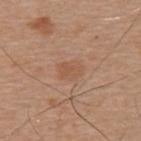follow-up: imaged on a skin check; not biopsied | patient: male, aged 63–67 | imaging modality: total-body-photography crop, ~15 mm field of view | illumination: white-light | TBP lesion metrics: a lesion area of about 5 mm² and an eccentricity of roughly 0.7; an average lesion color of about L≈54 a*≈21 b*≈32 (CIELAB), roughly 6 lightness units darker than nearby skin, and a normalized border contrast of about 5; a color-variation rating of about 2/10 and radial color variation of about 0.5; an automated nevus-likeness rating near 50 out of 100 and a lesion-detection confidence of about 100/100 | lesion size: ≈2.5 mm | location: the upper back.